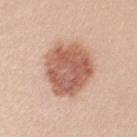Impression:
Imaged during a routine full-body skin examination; the lesion was not biopsied and no histopathology is available.
Image and clinical context:
The subject is a female roughly 25 years of age. A roughly 15 mm field-of-view crop from a total-body skin photograph. From the left upper arm.